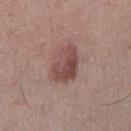Q: What are the patient's age and sex?
A: male, approximately 55 years of age
Q: What did automated image analysis measure?
A: a detector confidence of about 100 out of 100 that the crop contains a lesion
Q: How was the tile lit?
A: white-light illumination
Q: What is the anatomic site?
A: the right lower leg
Q: What kind of image is this?
A: ~15 mm crop, total-body skin-cancer survey
Q: How large is the lesion?
A: ~4.5 mm (longest diameter)